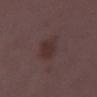Clinical impression:
The lesion was tiled from a total-body skin photograph and was not biopsied.
Image and clinical context:
A female patient aged around 35. A region of skin cropped from a whole-body photographic capture, roughly 15 mm wide. Approximately 4 mm at its widest. The lesion is located on the leg.